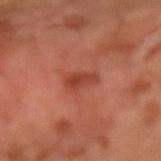Findings:
- notes: imaged on a skin check; not biopsied
- imaging modality: ~15 mm crop, total-body skin-cancer survey
- lesion size: ~3 mm (longest diameter)
- automated lesion analysis: a lesion color around L≈42 a*≈31 b*≈32 in CIELAB, a lesion–skin lightness drop of about 9, and a normalized lesion–skin contrast near 7; a classifier nevus-likeness of about 45/100 and a lesion-detection confidence of about 100/100
- patient: male, in their mid-60s